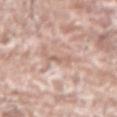This lesion was catalogued during total-body skin photography and was not selected for biopsy. A male patient, aged 73 to 77. A lesion tile, about 15 mm wide, cut from a 3D total-body photograph. The lesion is located on the left forearm.